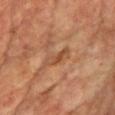The lesion was tiled from a total-body skin photograph and was not biopsied.
From the chest.
Imaged with cross-polarized lighting.
A region of skin cropped from a whole-body photographic capture, roughly 15 mm wide.
Measured at roughly 4 mm in maximum diameter.
A male patient, aged 58 to 62.
Automated image analysis of the tile measured border irregularity of about 3.5 on a 0–10 scale and a peripheral color-asymmetry measure near 1.